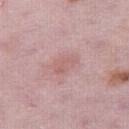Assessment:
The lesion was photographed on a routine skin check and not biopsied; there is no pathology result.
Image and clinical context:
The total-body-photography lesion software estimated an area of roughly 5 mm², an outline eccentricity of about 0.8 (0 = round, 1 = elongated), and two-axis asymmetry of about 0.25. The analysis additionally found a mean CIELAB color near L≈60 a*≈23 b*≈21. It also reported a border-irregularity index near 2.5/10, a within-lesion color-variation index near 1.5/10, and radial color variation of about 0.5. Captured under white-light illumination. A female subject roughly 30 years of age. The lesion's longest dimension is about 3 mm. From the leg. A close-up tile cropped from a whole-body skin photograph, about 15 mm across.